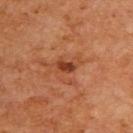{
  "biopsy_status": "not biopsied; imaged during a skin examination",
  "automated_metrics": {
    "area_mm2_approx": 2.5,
    "shape_asymmetry": 0.25,
    "nevus_likeness_0_100": 75
  },
  "patient": {
    "sex": "male",
    "age_approx": 60
  },
  "lighting": "cross-polarized",
  "site": "upper back",
  "lesion_size": {
    "long_diameter_mm_approx": 2.5
  },
  "image": {
    "source": "total-body photography crop",
    "field_of_view_mm": 15
  }
}